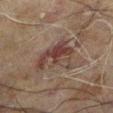patient: male, in their 60s
site: the left lower leg
image source: ~15 mm crop, total-body skin-cancer survey
image-analysis metrics: a border-irregularity rating of about 7/10 and a color-variation rating of about 6/10; a classifier nevus-likeness of about 10/100 and a detector confidence of about 100 out of 100 that the crop contains a lesion
lighting: cross-polarized illumination